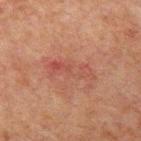{"biopsy_status": "not biopsied; imaged during a skin examination", "lesion_size": {"long_diameter_mm_approx": 5.5}, "patient": {"sex": "male", "age_approx": 60}, "automated_metrics": {"border_irregularity_0_10": 5.0, "peripheral_color_asymmetry": 2.0, "nevus_likeness_0_100": 0, "lesion_detection_confidence_0_100": 100}, "lighting": "cross-polarized", "image": {"source": "total-body photography crop", "field_of_view_mm": 15}, "site": "left upper arm"}